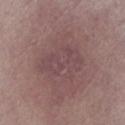A female patient aged approximately 60.
A lesion tile, about 15 mm wide, cut from a 3D total-body photograph.
Measured at roughly 5.5 mm in maximum diameter.
On the right lower leg.